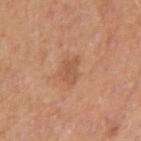biopsy status: total-body-photography surveillance lesion; no biopsy
subject: male, in their 40s
acquisition: ~15 mm crop, total-body skin-cancer survey
illumination: white-light illumination
location: the right upper arm
diameter: about 3 mm
automated metrics: a mean CIELAB color near L≈55 a*≈23 b*≈33